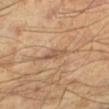follow-up: total-body-photography surveillance lesion; no biopsy
tile lighting: cross-polarized illumination
image: ~15 mm crop, total-body skin-cancer survey
lesion diameter: ≈3 mm
subject: male, in their mid-40s
body site: the left lower leg